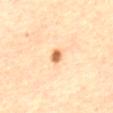Captured during whole-body skin photography for melanoma surveillance; the lesion was not biopsied.
From the abdomen.
The patient is a female about 65 years old.
Cropped from a total-body skin-imaging series; the visible field is about 15 mm.